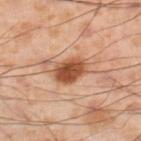This lesion was catalogued during total-body skin photography and was not selected for biopsy.
About 3.5 mm across.
The lesion is on the leg.
This is a cross-polarized tile.
This image is a 15 mm lesion crop taken from a total-body photograph.
A male subject aged around 55.